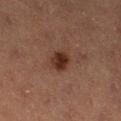Assessment:
The lesion was tiled from a total-body skin photograph and was not biopsied.
Acquisition and patient details:
The lesion is on the left lower leg. Cropped from a whole-body photographic skin survey; the tile spans about 15 mm. The recorded lesion diameter is about 3 mm. The patient is a female about 40 years old. Imaged with cross-polarized lighting. Automated tile analysis of the lesion measured a lesion color around L≈25 a*≈18 b*≈22 in CIELAB and a normalized border contrast of about 11. The software also gave a color-variation rating of about 3/10 and peripheral color asymmetry of about 1. The software also gave a nevus-likeness score of about 95/100 and lesion-presence confidence of about 100/100.